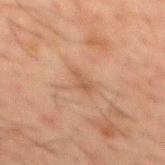Captured under cross-polarized illumination. A male patient, approximately 50 years of age. The lesion is located on the mid back. The lesion-visualizer software estimated an average lesion color of about L≈42 a*≈17 b*≈26 (CIELAB) and a normalized lesion–skin contrast near 5.5. And it measured border irregularity of about 4 on a 0–10 scale and internal color variation of about 0 on a 0–10 scale. Cropped from a total-body skin-imaging series; the visible field is about 15 mm.